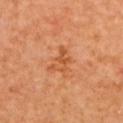<lesion>
<biopsy_status>not biopsied; imaged during a skin examination</biopsy_status>
<patient>
  <sex>female</sex>
</patient>
<image>
  <source>total-body photography crop</source>
  <field_of_view_mm>15</field_of_view_mm>
</image>
<lighting>cross-polarized</lighting>
<site>upper back</site>
<lesion_size>
  <long_diameter_mm_approx>3.5</long_diameter_mm_approx>
</lesion_size>
</lesion>